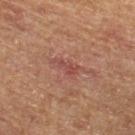Notes:
– notes · catalogued during a skin exam; not biopsied
– patient · male, in their mid- to late 60s
– anatomic site · the right lower leg
– imaging modality · total-body-photography crop, ~15 mm field of view